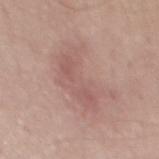workup — catalogued during a skin exam; not biopsied | size — about 7 mm | patient — male, aged approximately 65 | imaging modality — 15 mm crop, total-body photography | lighting — white-light | TBP lesion metrics — a within-lesion color-variation index near 3/10 | anatomic site — the mid back.